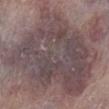biopsy status = imaged on a skin check; not biopsied
lesion size = about 17.5 mm
anatomic site = the leg
acquisition = ~15 mm crop, total-body skin-cancer survey
automated metrics = an area of roughly 115 mm², an eccentricity of roughly 0.7, and a symmetry-axis asymmetry near 0.4; a lesion color around L≈45 a*≈14 b*≈14 in CIELAB, roughly 10 lightness units darker than nearby skin, and a normalized border contrast of about 8.5; a border-irregularity rating of about 6.5/10 and a peripheral color-asymmetry measure near 1.5
illumination = white-light illumination
patient = female, in their mid-60s